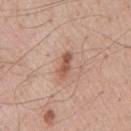notes: no biopsy performed (imaged during a skin exam)
subject: male, roughly 55 years of age
lighting: white-light
anatomic site: the chest
image source: ~15 mm crop, total-body skin-cancer survey
size: about 3.5 mm